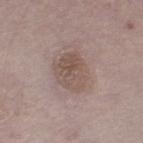The lesion was tiled from a total-body skin photograph and was not biopsied.
Located on the right thigh.
A male subject in their mid-70s.
A lesion tile, about 15 mm wide, cut from a 3D total-body photograph.